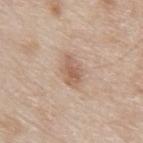{
  "biopsy_status": "not biopsied; imaged during a skin examination",
  "automated_metrics": {
    "cielab_L": 59,
    "cielab_a": 18,
    "cielab_b": 29,
    "vs_skin_darker_L": 10.0,
    "vs_skin_contrast_norm": 7.0,
    "border_irregularity_0_10": 2.5,
    "color_variation_0_10": 3.0,
    "nevus_likeness_0_100": 55,
    "lesion_detection_confidence_0_100": 100
  },
  "image": {
    "source": "total-body photography crop",
    "field_of_view_mm": 15
  },
  "lighting": "white-light",
  "site": "abdomen",
  "patient": {
    "sex": "male",
    "age_approx": 80
  }
}